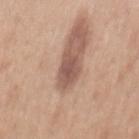Impression:
The lesion was tiled from a total-body skin photograph and was not biopsied.
Context:
This is a white-light tile. A roughly 15 mm field-of-view crop from a total-body skin photograph. Automated image analysis of the tile measured an automated nevus-likeness rating near 5 out of 100 and lesion-presence confidence of about 100/100. The lesion is located on the mid back. A female patient aged 38 to 42. The lesion's longest dimension is about 4 mm.